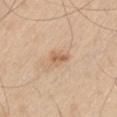Findings:
– anatomic site — the left thigh
– lighting — white-light illumination
– automated lesion analysis — an average lesion color of about L≈62 a*≈19 b*≈35 (CIELAB) and a normalized lesion–skin contrast near 6.5; border irregularity of about 3 on a 0–10 scale and a within-lesion color-variation index near 2/10
– subject — male, aged 58 to 62
– image source — total-body-photography crop, ~15 mm field of view